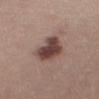The lesion was tiled from a total-body skin photograph and was not biopsied. The total-body-photography lesion software estimated a mean CIELAB color near L≈43 a*≈19 b*≈21, a lesion–skin lightness drop of about 15, and a normalized border contrast of about 11.5. And it measured a border-irregularity index near 3/10 and internal color variation of about 4 on a 0–10 scale. It also reported a nevus-likeness score of about 85/100 and lesion-presence confidence of about 100/100. A close-up tile cropped from a whole-body skin photograph, about 15 mm across. Located on the abdomen. The subject is a female roughly 40 years of age. The lesion's longest dimension is about 5 mm. Captured under white-light illumination.